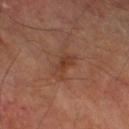follow-up: no biopsy performed (imaged during a skin exam)
image: 15 mm crop, total-body photography
tile lighting: cross-polarized
lesion diameter: about 3.5 mm
patient: male, in their 70s
image-analysis metrics: an average lesion color of about L≈38 a*≈22 b*≈29 (CIELAB) and a lesion–skin lightness drop of about 7; a border-irregularity index near 4.5/10 and a within-lesion color-variation index near 2.5/10; lesion-presence confidence of about 100/100
site: the right thigh